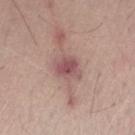Recorded during total-body skin imaging; not selected for excision or biopsy. A male patient roughly 65 years of age. A 15 mm close-up tile from a total-body photography series done for melanoma screening. This is a white-light tile. The lesion is on the left forearm. The recorded lesion diameter is about 3.5 mm.